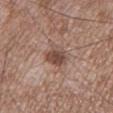{"biopsy_status": "not biopsied; imaged during a skin examination", "patient": {"sex": "male", "age_approx": 55}, "image": {"source": "total-body photography crop", "field_of_view_mm": 15}, "site": "left lower leg"}